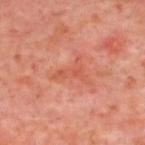Impression: Part of a total-body skin-imaging series; this lesion was reviewed on a skin check and was not flagged for biopsy. Image and clinical context: A roughly 15 mm field-of-view crop from a total-body skin photograph. The subject is in their mid-50s. Approximately 4 mm at its widest. This is a cross-polarized tile. The lesion is located on the upper back.